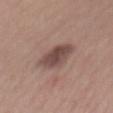notes: no biopsy performed (imaged during a skin exam) | imaging modality: ~15 mm crop, total-body skin-cancer survey | illumination: white-light illumination | automated metrics: an area of roughly 10 mm² and two-axis asymmetry of about 0.15; roughly 12 lightness units darker than nearby skin and a lesion-to-skin contrast of about 9 (normalized; higher = more distinct); peripheral color asymmetry of about 1.5; an automated nevus-likeness rating near 65 out of 100 | subject: female, aged 53 to 57 | size: about 5 mm | body site: the back.